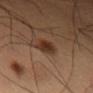Recorded during total-body skin imaging; not selected for excision or biopsy.
The lesion is on the left thigh.
A male patient, approximately 60 years of age.
Cropped from a total-body skin-imaging series; the visible field is about 15 mm.